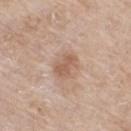Clinical impression: The lesion was tiled from a total-body skin photograph and was not biopsied. Acquisition and patient details: Automated tile analysis of the lesion measured an average lesion color of about L≈58 a*≈19 b*≈29 (CIELAB) and roughly 9 lightness units darker than nearby skin. And it measured border irregularity of about 2 on a 0–10 scale and a peripheral color-asymmetry measure near 1. And it measured a nevus-likeness score of about 5/100 and a lesion-detection confidence of about 100/100. Located on the right thigh. This is a white-light tile. A female patient in their mid- to late 70s. A roughly 15 mm field-of-view crop from a total-body skin photograph. The lesion's longest dimension is about 3 mm.